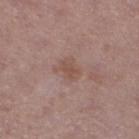Case summary:
* workup — total-body-photography surveillance lesion; no biopsy
* image source — total-body-photography crop, ~15 mm field of view
* size — ~3 mm (longest diameter)
* body site — the left lower leg
* image-analysis metrics — an eccentricity of roughly 0.65 and a symmetry-axis asymmetry near 0.3; an average lesion color of about L≈51 a*≈19 b*≈24 (CIELAB) and a normalized border contrast of about 5.5; a classifier nevus-likeness of about 0/100 and lesion-presence confidence of about 100/100
* subject — male, about 70 years old